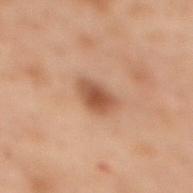This lesion was catalogued during total-body skin photography and was not selected for biopsy.
This is a cross-polarized tile.
Longest diameter approximately 3.5 mm.
A roughly 15 mm field-of-view crop from a total-body skin photograph.
On the back.
A female subject, approximately 55 years of age.